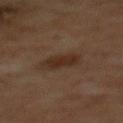{
  "biopsy_status": "not biopsied; imaged during a skin examination",
  "site": "chest",
  "lesion_size": {
    "long_diameter_mm_approx": 4.0
  },
  "patient": {
    "sex": "male",
    "age_approx": 60
  },
  "image": {
    "source": "total-body photography crop",
    "field_of_view_mm": 15
  },
  "lighting": "cross-polarized"
}